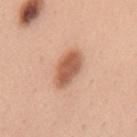The lesion was photographed on a routine skin check and not biopsied; there is no pathology result. A region of skin cropped from a whole-body photographic capture, roughly 15 mm wide. An algorithmic analysis of the crop reported an area of roughly 9.5 mm², an outline eccentricity of about 0.85 (0 = round, 1 = elongated), and a symmetry-axis asymmetry near 0.15. And it measured a border-irregularity rating of about 1.5/10, a color-variation rating of about 3/10, and radial color variation of about 1. And it measured a nevus-likeness score of about 100/100. On the back. The subject is a female approximately 35 years of age.